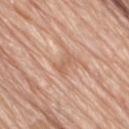No biopsy was performed on this lesion — it was imaged during a full skin examination and was not determined to be concerning.
This image is a 15 mm lesion crop taken from a total-body photograph.
About 2.5 mm across.
The lesion is on the right thigh.
A female patient about 75 years old.
Imaged with white-light lighting.
An algorithmic analysis of the crop reported an area of roughly 2.5 mm², a shape eccentricity near 0.9, and two-axis asymmetry of about 0.5.